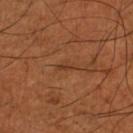No biopsy was performed on this lesion — it was imaged during a full skin examination and was not determined to be concerning.
This is a cross-polarized tile.
On the leg.
Automated tile analysis of the lesion measured a nevus-likeness score of about 0/100 and lesion-presence confidence of about 55/100.
The recorded lesion diameter is about 2.5 mm.
A roughly 15 mm field-of-view crop from a total-body skin photograph.
The subject is a male in their mid- to late 60s.